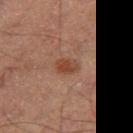  biopsy_status: not biopsied; imaged during a skin examination
  automated_metrics:
    area_mm2_approx: 5.0
    eccentricity: 0.75
    shape_asymmetry: 0.25
    cielab_L: 37
    cielab_a: 19
    cielab_b: 26
    vs_skin_contrast_norm: 7.5
  lesion_size:
    long_diameter_mm_approx: 2.5
  patient:
    sex: male
    age_approx: 65
  lighting: cross-polarized
  site: right thigh
  image:
    source: total-body photography crop
    field_of_view_mm: 15